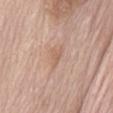Imaged during a routine full-body skin examination; the lesion was not biopsied and no histopathology is available.
The lesion is located on the abdomen.
A male patient aged approximately 70.
A roughly 15 mm field-of-view crop from a total-body skin photograph.